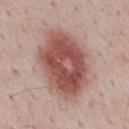follow-up = total-body-photography surveillance lesion; no biopsy